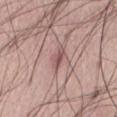This lesion was catalogued during total-body skin photography and was not selected for biopsy.
A male subject, in their mid- to late 70s.
Automated image analysis of the tile measured an outline eccentricity of about 0.85 (0 = round, 1 = elongated) and two-axis asymmetry of about 0.3. It also reported border irregularity of about 3 on a 0–10 scale, internal color variation of about 3 on a 0–10 scale, and radial color variation of about 1. The analysis additionally found a nevus-likeness score of about 0/100 and a detector confidence of about 95 out of 100 that the crop contains a lesion.
On the abdomen.
The lesion's longest dimension is about 3 mm.
This image is a 15 mm lesion crop taken from a total-body photograph.